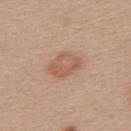Part of a total-body skin-imaging series; this lesion was reviewed on a skin check and was not flagged for biopsy.